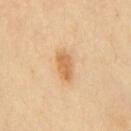Q: What lighting was used for the tile?
A: cross-polarized
Q: What is the imaging modality?
A: ~15 mm tile from a whole-body skin photo
Q: What is the anatomic site?
A: the chest
Q: Patient demographics?
A: male, roughly 40 years of age
Q: What is the lesion's diameter?
A: about 4 mm